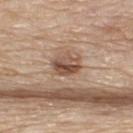Recorded during total-body skin imaging; not selected for excision or biopsy.